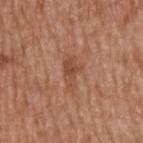The lesion was tiled from a total-body skin photograph and was not biopsied. The subject is a male aged around 65. Captured under white-light illumination. Automated image analysis of the tile measured a footprint of about 5.5 mm², an eccentricity of roughly 0.85, and a symmetry-axis asymmetry near 0.35. A roughly 15 mm field-of-view crop from a total-body skin photograph. Measured at roughly 4 mm in maximum diameter. The lesion is located on the back.